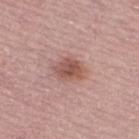The lesion was tiled from a total-body skin photograph and was not biopsied.
The lesion is on the right thigh.
This image is a 15 mm lesion crop taken from a total-body photograph.
The tile uses white-light illumination.
The subject is a female approximately 60 years of age.